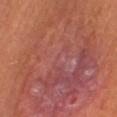Case summary:
- workup — imaged on a skin check; not biopsied
- size — ~23.5 mm (longest diameter)
- imaging modality — total-body-photography crop, ~15 mm field of view
- subject — female, in their 50s
- automated lesion analysis — an average lesion color of about L≈49 a*≈27 b*≈27 (CIELAB) and a lesion–skin lightness drop of about 6; a nevus-likeness score of about 0/100 and a detector confidence of about 90 out of 100 that the crop contains a lesion